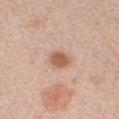<case>
<biopsy_status>not biopsied; imaged during a skin examination</biopsy_status>
<site>chest</site>
<patient>
  <sex>female</sex>
  <age_approx>40</age_approx>
</patient>
<automated_metrics>
  <area_mm2_approx>5.0</area_mm2_approx>
  <shape_asymmetry>0.2</shape_asymmetry>
  <cielab_L>59</cielab_L>
  <cielab_a>21</cielab_a>
  <cielab_b>32</cielab_b>
  <vs_skin_darker_L>12.0</vs_skin_darker_L>
  <vs_skin_contrast_norm>8.5</vs_skin_contrast_norm>
  <color_variation_0_10>2.0</color_variation_0_10>
  <peripheral_color_asymmetry>0.5</peripheral_color_asymmetry>
</automated_metrics>
<image>
  <source>total-body photography crop</source>
  <field_of_view_mm>15</field_of_view_mm>
</image>
</case>